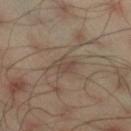Assessment:
The lesion was photographed on a routine skin check and not biopsied; there is no pathology result.
Background:
A close-up tile cropped from a whole-body skin photograph, about 15 mm across. A male patient, about 45 years old. Longest diameter approximately 2.5 mm. The tile uses cross-polarized illumination. The lesion is on the right thigh. The total-body-photography lesion software estimated a lesion area of about 4.5 mm² and a shape-asymmetry score of about 0.35 (0 = symmetric). It also reported an automated nevus-likeness rating near 0 out of 100 and lesion-presence confidence of about 100/100.